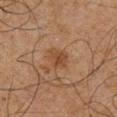Q: Is there a histopathology result?
A: total-body-photography surveillance lesion; no biopsy
Q: What are the patient's age and sex?
A: male, roughly 65 years of age
Q: What is the anatomic site?
A: the right lower leg
Q: What kind of image is this?
A: ~15 mm crop, total-body skin-cancer survey
Q: What lighting was used for the tile?
A: cross-polarized